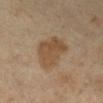No biopsy was performed on this lesion — it was imaged during a full skin examination and was not determined to be concerning. A female subject, roughly 40 years of age. The total-body-photography lesion software estimated an area of roughly 15 mm², a shape eccentricity near 0.7, and a symmetry-axis asymmetry near 0.25. The software also gave a mean CIELAB color near L≈41 a*≈13 b*≈28, roughly 7 lightness units darker than nearby skin, and a normalized lesion–skin contrast near 7. The software also gave a nevus-likeness score of about 5/100 and a lesion-detection confidence of about 100/100. The recorded lesion diameter is about 5 mm. On the right lower leg. A 15 mm close-up extracted from a 3D total-body photography capture.